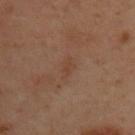workup: total-body-photography surveillance lesion; no biopsy
diameter: about 2.5 mm
illumination: cross-polarized illumination
automated metrics: a lesion color around L≈41 a*≈19 b*≈28 in CIELAB, about 5 CIELAB-L* units darker than the surrounding skin, and a normalized lesion–skin contrast near 4.5
patient: male, aged 48 to 52
imaging modality: 15 mm crop, total-body photography
body site: the upper back